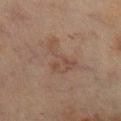Q: How was this image acquired?
A: ~15 mm crop, total-body skin-cancer survey
Q: How large is the lesion?
A: ~5 mm (longest diameter)
Q: Automated lesion metrics?
A: border irregularity of about 7.5 on a 0–10 scale, internal color variation of about 3 on a 0–10 scale, and a peripheral color-asymmetry measure near 1; a classifier nevus-likeness of about 0/100 and a lesion-detection confidence of about 100/100
Q: What are the patient's age and sex?
A: female, aged 53 to 57
Q: What is the anatomic site?
A: the right lower leg